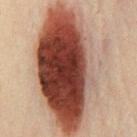notes: no biopsy performed (imaged during a skin exam)
size: about 15.5 mm
patient: male, in their 50s
imaging modality: ~15 mm crop, total-body skin-cancer survey
body site: the chest
tile lighting: cross-polarized illumination